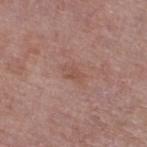Q: Was this lesion biopsied?
A: total-body-photography surveillance lesion; no biopsy
Q: What are the patient's age and sex?
A: male, aged 73 to 77
Q: How large is the lesion?
A: about 2.5 mm
Q: Illumination type?
A: white-light illumination
Q: Lesion location?
A: the right thigh
Q: What is the imaging modality?
A: total-body-photography crop, ~15 mm field of view